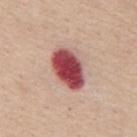biopsy status = catalogued during a skin exam; not biopsied | illumination = white-light illumination | image source = ~15 mm crop, total-body skin-cancer survey | subject = male, approximately 65 years of age | location = the back | lesion size = ≈5.5 mm.